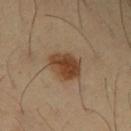Findings:
– follow-up — imaged on a skin check; not biopsied
– image source — 15 mm crop, total-body photography
– body site — the right upper arm
– subject — male, aged around 40
– lighting — cross-polarized illumination